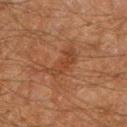{"biopsy_status": "not biopsied; imaged during a skin examination", "patient": {"sex": "male", "age_approx": 60}, "site": "left lower leg", "image": {"source": "total-body photography crop", "field_of_view_mm": 15}}